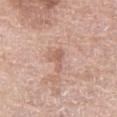patient:
  sex: female
  age_approx: 60
image:
  source: total-body photography crop
  field_of_view_mm: 15
lesion_size:
  long_diameter_mm_approx: 3.0
site: right thigh
lighting: white-light